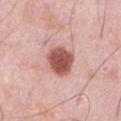Q: Lesion location?
A: the abdomen
Q: Lesion size?
A: about 4 mm
Q: What kind of image is this?
A: 15 mm crop, total-body photography
Q: Illumination type?
A: white-light illumination
Q: Patient demographics?
A: male, aged around 55